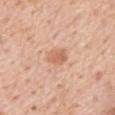Imaged during a routine full-body skin examination; the lesion was not biopsied and no histopathology is available. The patient is a male in their 60s. About 3 mm across. Imaged with white-light lighting. The lesion is on the mid back. Cropped from a total-body skin-imaging series; the visible field is about 15 mm.